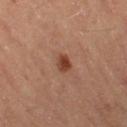Case summary:
- notes: no biopsy performed (imaged during a skin exam)
- location: the left thigh
- illumination: cross-polarized
- acquisition: ~15 mm tile from a whole-body skin photo
- patient: female, approximately 45 years of age
- lesion diameter: about 2.5 mm
- automated metrics: roughly 11 lightness units darker than nearby skin and a lesion-to-skin contrast of about 9 (normalized; higher = more distinct); a lesion-detection confidence of about 100/100